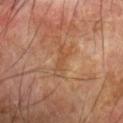Clinical impression:
Recorded during total-body skin imaging; not selected for excision or biopsy.
Context:
About 4 mm across. From the left forearm. A roughly 15 mm field-of-view crop from a total-body skin photograph. Captured under cross-polarized illumination. A male subject aged 68–72.